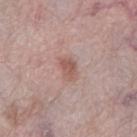<lesion>
  <biopsy_status>not biopsied; imaged during a skin examination</biopsy_status>
</lesion>